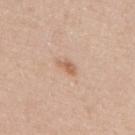{"biopsy_status": "not biopsied; imaged during a skin examination", "site": "upper back", "image": {"source": "total-body photography crop", "field_of_view_mm": 15}, "lesion_size": {"long_diameter_mm_approx": 3.0}, "patient": {"sex": "female", "age_approx": 40}, "lighting": "white-light"}